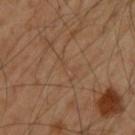The lesion was photographed on a routine skin check and not biopsied; there is no pathology result. The lesion is located on the upper back. A male patient, about 55 years old. Automated image analysis of the tile measured an average lesion color of about L≈43 a*≈16 b*≈29 (CIELAB) and about 3 CIELAB-L* units darker than the surrounding skin. The software also gave a border-irregularity index near 6.5/10, internal color variation of about 0 on a 0–10 scale, and peripheral color asymmetry of about 0. The software also gave a classifier nevus-likeness of about 0/100. Measured at roughly 3.5 mm in maximum diameter. A 15 mm close-up tile from a total-body photography series done for melanoma screening. The tile uses cross-polarized illumination.